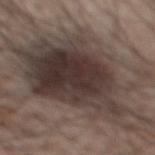Image and clinical context: This is a white-light tile. A male patient in their 60s. Cropped from a total-body skin-imaging series; the visible field is about 15 mm. The lesion-visualizer software estimated a lesion area of about 75 mm², an eccentricity of roughly 0.85, and a shape-asymmetry score of about 0.4 (0 = symmetric). And it measured an average lesion color of about L≈37 a*≈11 b*≈17 (CIELAB), a lesion–skin lightness drop of about 12, and a lesion-to-skin contrast of about 11 (normalized; higher = more distinct). The analysis additionally found a border-irregularity rating of about 8/10, internal color variation of about 7 on a 0–10 scale, and radial color variation of about 2. The analysis additionally found a lesion-detection confidence of about 85/100. Measured at roughly 15 mm in maximum diameter. On the back.